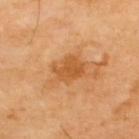Clinical impression: This lesion was catalogued during total-body skin photography and was not selected for biopsy. Image and clinical context: On the upper back. The subject is a male aged 68 to 72. A region of skin cropped from a whole-body photographic capture, roughly 15 mm wide. Imaged with cross-polarized lighting.